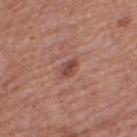Case summary:
– follow-up: no biopsy performed (imaged during a skin exam)
– subject: female, about 60 years old
– tile lighting: white-light
– body site: the leg
– imaging modality: ~15 mm tile from a whole-body skin photo